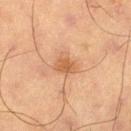Clinical impression:
Part of a total-body skin-imaging series; this lesion was reviewed on a skin check and was not flagged for biopsy.